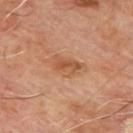Imaged during a routine full-body skin examination; the lesion was not biopsied and no histopathology is available.
The subject is a male roughly 65 years of age.
A 15 mm close-up tile from a total-body photography series done for melanoma screening.
Captured under cross-polarized illumination.
Approximately 4.5 mm at its widest.
The lesion is on the upper back.